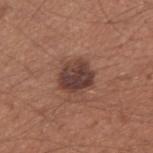The tile uses white-light illumination.
A close-up tile cropped from a whole-body skin photograph, about 15 mm across.
The lesion's longest dimension is about 3.5 mm.
The lesion is on the right upper arm.
A male subject, aged 33–37.